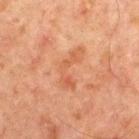Part of a total-body skin-imaging series; this lesion was reviewed on a skin check and was not flagged for biopsy.
On the chest.
The lesion-visualizer software estimated an area of roughly 7.5 mm², an outline eccentricity of about 0.9 (0 = round, 1 = elongated), and a symmetry-axis asymmetry near 0.65. It also reported a mean CIELAB color near L≈47 a*≈23 b*≈31, about 6 CIELAB-L* units darker than the surrounding skin, and a normalized lesion–skin contrast near 5. And it measured a border-irregularity rating of about 8/10, a within-lesion color-variation index near 3.5/10, and peripheral color asymmetry of about 1. And it measured a nevus-likeness score of about 0/100.
A male subject, aged 63–67.
About 5 mm across.
This image is a 15 mm lesion crop taken from a total-body photograph.
Imaged with cross-polarized lighting.